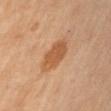follow-up = imaged on a skin check; not biopsied.